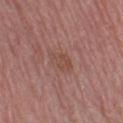{
  "biopsy_status": "not biopsied; imaged during a skin examination",
  "patient": {
    "sex": "female",
    "age_approx": 55
  },
  "image": {
    "source": "total-body photography crop",
    "field_of_view_mm": 15
  },
  "lesion_size": {
    "long_diameter_mm_approx": 3.5
  },
  "automated_metrics": {
    "area_mm2_approx": 5.0,
    "eccentricity": 0.8,
    "shape_asymmetry": 0.2,
    "border_irregularity_0_10": 2.0,
    "color_variation_0_10": 2.5,
    "lesion_detection_confidence_0_100": 100
  },
  "site": "left lower leg"
}